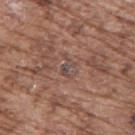Impression: Recorded during total-body skin imaging; not selected for excision or biopsy. Clinical summary: Approximately 3 mm at its widest. On the upper back. The patient is a male aged around 75. A 15 mm crop from a total-body photograph taken for skin-cancer surveillance. The total-body-photography lesion software estimated a lesion area of about 3.5 mm², a shape eccentricity near 0.8, and two-axis asymmetry of about 0.55. The analysis additionally found a within-lesion color-variation index near 0/10 and radial color variation of about 0. And it measured an automated nevus-likeness rating near 0 out of 100. Imaged with white-light lighting.